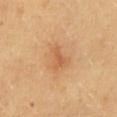| feature | finding |
|---|---|
| size | about 3 mm |
| lighting | cross-polarized illumination |
| subject | female, aged 43–47 |
| anatomic site | the mid back |
| imaging modality | 15 mm crop, total-body photography |